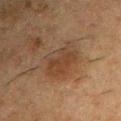Assessment:
The lesion was photographed on a routine skin check and not biopsied; there is no pathology result.
Background:
Automated image analysis of the tile measured an area of roughly 14 mm², a shape eccentricity near 0.55, and a shape-asymmetry score of about 0.25 (0 = symmetric). The software also gave a detector confidence of about 100 out of 100 that the crop contains a lesion. On the right upper arm. The tile uses cross-polarized illumination. The patient is a male aged around 50. A 15 mm close-up tile from a total-body photography series done for melanoma screening.